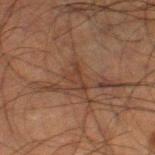Assessment: Recorded during total-body skin imaging; not selected for excision or biopsy. Background: Automated tile analysis of the lesion measured border irregularity of about 5.5 on a 0–10 scale and peripheral color asymmetry of about 0. A close-up tile cropped from a whole-body skin photograph, about 15 mm across. From the leg. Imaged with cross-polarized lighting. A male patient about 50 years old. About 3 mm across.